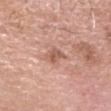Notes:
* follow-up: total-body-photography surveillance lesion; no biopsy
* anatomic site: the head or neck
* lesion size: about 3 mm
* patient: male, approximately 70 years of age
* TBP lesion metrics: a shape eccentricity near 0.85; a border-irregularity index near 3/10 and a color-variation rating of about 2.5/10; an automated nevus-likeness rating near 0 out of 100 and a lesion-detection confidence of about 100/100
* image: 15 mm crop, total-body photography
* illumination: white-light illumination